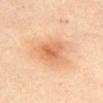workup = no biopsy performed (imaged during a skin exam) | subject = female, aged around 35 | automated lesion analysis = a shape eccentricity near 0.55 and a symmetry-axis asymmetry near 0.3; a border-irregularity rating of about 3/10 and a within-lesion color-variation index near 3.5/10; an automated nevus-likeness rating near 55 out of 100 and a detector confidence of about 100 out of 100 that the crop contains a lesion | anatomic site = the abdomen | illumination = cross-polarized illumination | imaging modality = ~15 mm crop, total-body skin-cancer survey.